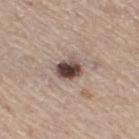This lesion was catalogued during total-body skin photography and was not selected for biopsy. An algorithmic analysis of the crop reported a lesion area of about 6.5 mm², an outline eccentricity of about 0.55 (0 = round, 1 = elongated), and a symmetry-axis asymmetry near 0.2. It also reported a lesion color around L≈45 a*≈15 b*≈20 in CIELAB and a lesion-to-skin contrast of about 13 (normalized; higher = more distinct). And it measured border irregularity of about 2 on a 0–10 scale and a color-variation rating of about 8/10. Located on the leg. A female patient, approximately 70 years of age. The tile uses white-light illumination. A 15 mm crop from a total-body photograph taken for skin-cancer surveillance. Approximately 3 mm at its widest.